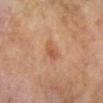- workup — catalogued during a skin exam; not biopsied
- TBP lesion metrics — roughly 8 lightness units darker than nearby skin and a normalized lesion–skin contrast near 6; a border-irregularity index near 2.5/10, a color-variation rating of about 2/10, and peripheral color asymmetry of about 0.5
- patient — male, roughly 65 years of age
- lesion diameter — ~2.5 mm (longest diameter)
- tile lighting — cross-polarized
- image — total-body-photography crop, ~15 mm field of view